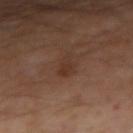Impression:
The lesion was photographed on a routine skin check and not biopsied; there is no pathology result.